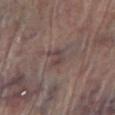Located on the leg. Automated image analysis of the tile measured a shape eccentricity near 0.6 and a symmetry-axis asymmetry near 0.35. And it measured a lesion color around L≈42 a*≈15 b*≈16 in CIELAB, about 6 CIELAB-L* units darker than the surrounding skin, and a normalized lesion–skin contrast near 6. A 15 mm close-up extracted from a 3D total-body photography capture. Longest diameter approximately 2.5 mm. The subject is a male aged 68 to 72.